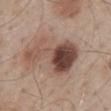Impression: Part of a total-body skin-imaging series; this lesion was reviewed on a skin check and was not flagged for biopsy. Image and clinical context: On the back. The tile uses white-light illumination. The subject is a male in their mid- to late 50s. A 15 mm close-up tile from a total-body photography series done for melanoma screening. The total-body-photography lesion software estimated a border-irregularity index near 7.5/10, internal color variation of about 9 on a 0–10 scale, and peripheral color asymmetry of about 3.